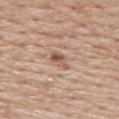{
  "biopsy_status": "not biopsied; imaged during a skin examination",
  "image": {
    "source": "total-body photography crop",
    "field_of_view_mm": 15
  },
  "site": "upper back",
  "automated_metrics": {
    "nevus_likeness_0_100": 0,
    "lesion_detection_confidence_0_100": 100
  },
  "patient": {
    "sex": "male",
    "age_approx": 60
  }
}